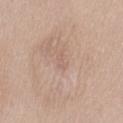Imaged with white-light lighting. From the lower back. A female patient, aged approximately 25. Approximately 1.5 mm at its widest. Cropped from a whole-body photographic skin survey; the tile spans about 15 mm.